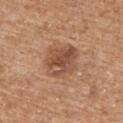<record>
  <biopsy_status>not biopsied; imaged during a skin examination</biopsy_status>
  <image>
    <source>total-body photography crop</source>
    <field_of_view_mm>15</field_of_view_mm>
  </image>
  <site>upper back</site>
  <lesion_size>
    <long_diameter_mm_approx>4.0</long_diameter_mm_approx>
  </lesion_size>
  <patient>
    <sex>female</sex>
    <age_approx>70</age_approx>
  </patient>
  <automated_metrics>
    <area_mm2_approx>13.0</area_mm2_approx>
    <eccentricity>0.4</eccentricity>
    <shape_asymmetry>0.15</shape_asymmetry>
    <cielab_L>50</cielab_L>
    <cielab_a>22</cielab_a>
    <cielab_b>31</cielab_b>
    <vs_skin_darker_L>10.0</vs_skin_darker_L>
    <vs_skin_contrast_norm>7.5</vs_skin_contrast_norm>
    <border_irregularity_0_10>2.0</border_irregularity_0_10>
    <color_variation_0_10>5.5</color_variation_0_10>
    <peripheral_color_asymmetry>2.0</peripheral_color_asymmetry>
    <nevus_likeness_0_100>30</nevus_likeness_0_100>
  </automated_metrics>
  <lighting>white-light</lighting>
</record>